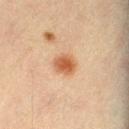biopsy status = no biopsy performed (imaged during a skin exam)
patient = male, aged approximately 40
lesion diameter = ≈3 mm
image = ~15 mm crop, total-body skin-cancer survey
site = the left thigh